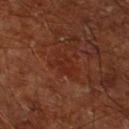• follow-up: imaged on a skin check; not biopsied
• body site: the left lower leg
• TBP lesion metrics: an average lesion color of about L≈26 a*≈25 b*≈27 (CIELAB), roughly 4 lightness units darker than nearby skin, and a normalized lesion–skin contrast near 4.5; a border-irregularity rating of about 2.5/10 and a within-lesion color-variation index near 1.5/10
• patient: male, aged around 65
• diameter: about 3 mm
• imaging modality: 15 mm crop, total-body photography